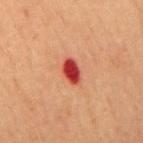<case>
<biopsy_status>not biopsied; imaged during a skin examination</biopsy_status>
<patient>
  <sex>male</sex>
  <age_approx>65</age_approx>
</patient>
<site>mid back</site>
<image>
  <source>total-body photography crop</source>
  <field_of_view_mm>15</field_of_view_mm>
</image>
<lesion_size>
  <long_diameter_mm_approx>3.0</long_diameter_mm_approx>
</lesion_size>
<lighting>cross-polarized</lighting>
</case>